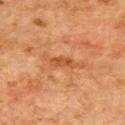{"biopsy_status": "not biopsied; imaged during a skin examination", "site": "front of the torso", "lighting": "cross-polarized", "patient": {"sex": "male", "age_approx": 80}, "image": {"source": "total-body photography crop", "field_of_view_mm": 15}}